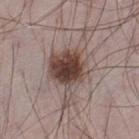Q: Was a biopsy performed?
A: no biopsy performed (imaged during a skin exam)
Q: How was the tile lit?
A: white-light
Q: How was this image acquired?
A: ~15 mm tile from a whole-body skin photo
Q: How large is the lesion?
A: ≈9 mm
Q: Who is the patient?
A: male, approximately 70 years of age
Q: Automated lesion metrics?
A: an average lesion color of about L≈46 a*≈15 b*≈21 (CIELAB), roughly 14 lightness units darker than nearby skin, and a normalized lesion–skin contrast near 10.5; a classifier nevus-likeness of about 100/100 and lesion-presence confidence of about 100/100
Q: Lesion location?
A: the left thigh